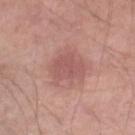Notes:
* workup: imaged on a skin check; not biopsied
* image: ~15 mm tile from a whole-body skin photo
* size: ≈4 mm
* tile lighting: white-light illumination
* subject: male, in their mid-50s
* body site: the leg